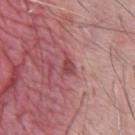Imaged during a routine full-body skin examination; the lesion was not biopsied and no histopathology is available.
The tile uses white-light illumination.
This image is a 15 mm lesion crop taken from a total-body photograph.
The lesion is on the chest.
The patient is a male approximately 60 years of age.
About 3 mm across.